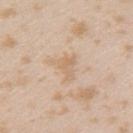biopsy status: total-body-photography surveillance lesion; no biopsy | lighting: white-light illumination | site: the left upper arm | imaging modality: ~15 mm tile from a whole-body skin photo | patient: female, approximately 25 years of age.